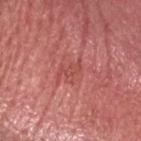  biopsy_status: not biopsied; imaged during a skin examination
  automated_metrics:
    peripheral_color_asymmetry: 0.0
  site: head or neck
  lesion_size:
    long_diameter_mm_approx: 3.0
  image:
    source: total-body photography crop
    field_of_view_mm: 15
  lighting: white-light
  patient:
    sex: male
    age_approx: 75